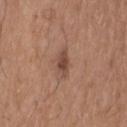The lesion is on the head or neck.
A region of skin cropped from a whole-body photographic capture, roughly 15 mm wide.
A male patient in their 70s.
Measured at roughly 3.5 mm in maximum diameter.
Imaged with white-light lighting.